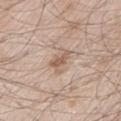This lesion was catalogued during total-body skin photography and was not selected for biopsy. A 15 mm crop from a total-body photograph taken for skin-cancer surveillance. The lesion's longest dimension is about 3.5 mm. On the left lower leg. Imaged with white-light lighting. A male subject, aged 58–62.